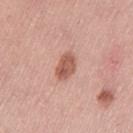Imaged during a routine full-body skin examination; the lesion was not biopsied and no histopathology is available.
A female subject, in their 40s.
This is a white-light tile.
A 15 mm close-up extracted from a 3D total-body photography capture.
Longest diameter approximately 3.5 mm.
The lesion is on the left thigh.